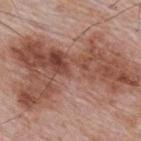– workup · catalogued during a skin exam; not biopsied
– lesion diameter · ≈15.5 mm
– imaging modality · ~15 mm crop, total-body skin-cancer survey
– site · the upper back
– tile lighting · white-light
– patient · male, aged around 65